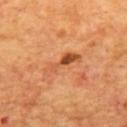lesion_size:
  long_diameter_mm_approx: 5.0
site: upper back
image:
  source: total-body photography crop
  field_of_view_mm: 15
patient:
  sex: male
  age_approx: 65
automated_metrics:
  shape_asymmetry: 0.35
  border_irregularity_0_10: 5.0
  color_variation_0_10: 5.0
  peripheral_color_asymmetry: 1.0
lighting: cross-polarized